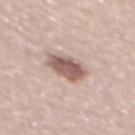The lesion was photographed on a routine skin check and not biopsied; there is no pathology result.